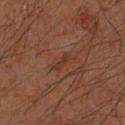{"biopsy_status": "not biopsied; imaged during a skin examination", "patient": {"sex": "male", "age_approx": 65}, "image": {"source": "total-body photography crop", "field_of_view_mm": 15}, "site": "left forearm", "lighting": "cross-polarized"}